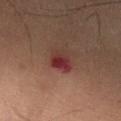Q: Is there a histopathology result?
A: imaged on a skin check; not biopsied
Q: What kind of image is this?
A: total-body-photography crop, ~15 mm field of view
Q: Who is the patient?
A: male, about 50 years old
Q: Where on the body is the lesion?
A: the right lower leg
Q: How large is the lesion?
A: about 3 mm
Q: How was the tile lit?
A: cross-polarized